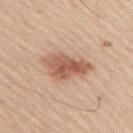This lesion was catalogued during total-body skin photography and was not selected for biopsy.
The tile uses white-light illumination.
The recorded lesion diameter is about 6 mm.
A male subject in their mid-40s.
An algorithmic analysis of the crop reported a mean CIELAB color near L≈58 a*≈22 b*≈31, about 13 CIELAB-L* units darker than the surrounding skin, and a lesion-to-skin contrast of about 8.5 (normalized; higher = more distinct).
The lesion is on the upper back.
A 15 mm close-up tile from a total-body photography series done for melanoma screening.